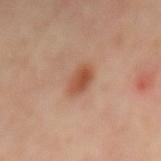The tile uses cross-polarized illumination. Longest diameter approximately 3.5 mm. A male subject aged around 55. Cropped from a whole-body photographic skin survey; the tile spans about 15 mm. From the mid back.